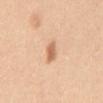Recorded during total-body skin imaging; not selected for excision or biopsy.
Imaged with white-light lighting.
A 15 mm close-up tile from a total-body photography series done for melanoma screening.
A female subject, aged around 30.
The lesion is located on the front of the torso.
Longest diameter approximately 2.5 mm.